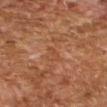Imaged during a routine full-body skin examination; the lesion was not biopsied and no histopathology is available. The patient is a male approximately 70 years of age. A 15 mm crop from a total-body photograph taken for skin-cancer surveillance. Imaged with cross-polarized lighting. About 2.5 mm across. Located on the arm.